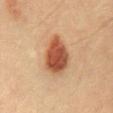Imaged during a routine full-body skin examination; the lesion was not biopsied and no histopathology is available. Measured at roughly 5 mm in maximum diameter. Cropped from a total-body skin-imaging series; the visible field is about 15 mm. The patient is a male aged 38–42. From the lower back. Imaged with cross-polarized lighting.